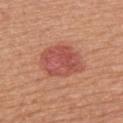Notes:
- workup: catalogued during a skin exam; not biopsied
- patient: male, aged 53 to 57
- tile lighting: white-light illumination
- image source: 15 mm crop, total-body photography
- TBP lesion metrics: a footprint of about 17 mm² and an eccentricity of roughly 0.7; an average lesion color of about L≈53 a*≈30 b*≈29 (CIELAB), about 10 CIELAB-L* units darker than the surrounding skin, and a normalized lesion–skin contrast near 7.5; a classifier nevus-likeness of about 100/100 and lesion-presence confidence of about 100/100
- size: about 5.5 mm
- location: the upper back